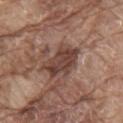Q: Was a biopsy performed?
A: total-body-photography surveillance lesion; no biopsy
Q: Lesion size?
A: ≈5 mm
Q: Automated lesion metrics?
A: a footprint of about 12 mm², an eccentricity of roughly 0.8, and a symmetry-axis asymmetry near 0.35; an average lesion color of about L≈42 a*≈19 b*≈24 (CIELAB), roughly 12 lightness units darker than nearby skin, and a normalized border contrast of about 9; border irregularity of about 4 on a 0–10 scale; a classifier nevus-likeness of about 65/100 and a lesion-detection confidence of about 100/100
Q: Where on the body is the lesion?
A: the mid back
Q: Illumination type?
A: white-light illumination
Q: What kind of image is this?
A: 15 mm crop, total-body photography
Q: Patient demographics?
A: male, approximately 80 years of age